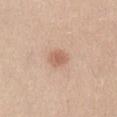Imaged during a routine full-body skin examination; the lesion was not biopsied and no histopathology is available. The patient is a female aged 23–27. Automated image analysis of the tile measured a lesion-to-skin contrast of about 6.5 (normalized; higher = more distinct). It also reported a border-irregularity index near 1/10, a within-lesion color-variation index near 2/10, and radial color variation of about 0.5. From the abdomen. Approximately 2.5 mm at its widest. A 15 mm close-up tile from a total-body photography series done for melanoma screening.